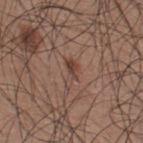{
  "biopsy_status": "not biopsied; imaged during a skin examination",
  "patient": {
    "sex": "male",
    "age_approx": 35
  },
  "lesion_size": {
    "long_diameter_mm_approx": 3.5
  },
  "site": "upper back",
  "lighting": "white-light",
  "image": {
    "source": "total-body photography crop",
    "field_of_view_mm": 15
  }
}